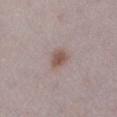Notes:
* follow-up — imaged on a skin check; not biopsied
* location — the left lower leg
* image source — 15 mm crop, total-body photography
* tile lighting — white-light
* lesion size — ≈2.5 mm
* automated metrics — a footprint of about 4 mm², an outline eccentricity of about 0.65 (0 = round, 1 = elongated), and a symmetry-axis asymmetry near 0.2; a classifier nevus-likeness of about 80/100 and a detector confidence of about 100 out of 100 that the crop contains a lesion
* patient — female, aged around 30